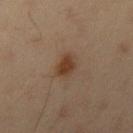The lesion was photographed on a routine skin check and not biopsied; there is no pathology result. This is a cross-polarized tile. Located on the right upper arm. The subject is a male aged around 35. Approximately 2.5 mm at its widest. A 15 mm close-up tile from a total-body photography series done for melanoma screening.